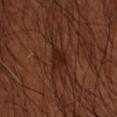Case summary:
– notes · catalogued during a skin exam; not biopsied
– lighting · cross-polarized
– lesion diameter · about 3 mm
– subject · male, approximately 50 years of age
– image · 15 mm crop, total-body photography
– anatomic site · the left forearm